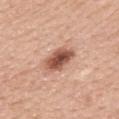The lesion was tiled from a total-body skin photograph and was not biopsied.
The subject is a female roughly 60 years of age.
Approximately 4.5 mm at its widest.
An algorithmic analysis of the crop reported a border-irregularity index near 2.5/10, a within-lesion color-variation index near 6/10, and peripheral color asymmetry of about 2. It also reported an automated nevus-likeness rating near 95 out of 100.
The lesion is located on the upper back.
Cropped from a total-body skin-imaging series; the visible field is about 15 mm.